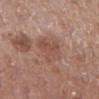• biopsy status: no biopsy performed (imaged during a skin exam)
• site: the right lower leg
• imaging modality: ~15 mm tile from a whole-body skin photo
• patient: female, aged around 75
• tile lighting: white-light